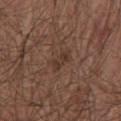Imaged during a routine full-body skin examination; the lesion was not biopsied and no histopathology is available. The total-body-photography lesion software estimated a lesion color around L≈35 a*≈17 b*≈23 in CIELAB, a lesion–skin lightness drop of about 7, and a normalized border contrast of about 6.5. A 15 mm close-up extracted from a 3D total-body photography capture. Longest diameter approximately 3 mm. The patient is a male aged 63 to 67. On the chest. This is a white-light tile.